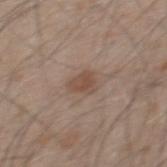This lesion was catalogued during total-body skin photography and was not selected for biopsy.
On the mid back.
This image is a 15 mm lesion crop taken from a total-body photograph.
The patient is a male aged 48 to 52.
The total-body-photography lesion software estimated an area of roughly 4.5 mm². The software also gave a border-irregularity index near 3/10. The analysis additionally found an automated nevus-likeness rating near 60 out of 100.
The tile uses white-light illumination.